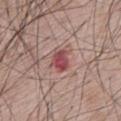workup — catalogued during a skin exam; not biopsied | lesion diameter — about 3 mm | image — 15 mm crop, total-body photography | patient — male, in their mid-60s | body site — the mid back.